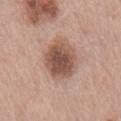Q: Was a biopsy performed?
A: catalogued during a skin exam; not biopsied
Q: How was this image acquired?
A: ~15 mm tile from a whole-body skin photo
Q: How large is the lesion?
A: ~5.5 mm (longest diameter)
Q: Where on the body is the lesion?
A: the mid back
Q: What did automated image analysis measure?
A: an area of roughly 16 mm², an outline eccentricity of about 0.65 (0 = round, 1 = elongated), and a shape-asymmetry score of about 0.1 (0 = symmetric); a border-irregularity rating of about 1.5/10 and radial color variation of about 1
Q: How was the tile lit?
A: white-light illumination
Q: Patient demographics?
A: male, aged 73–77